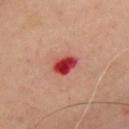Assessment:
Imaged during a routine full-body skin examination; the lesion was not biopsied and no histopathology is available.
Clinical summary:
A male patient, approximately 50 years of age. The lesion-visualizer software estimated border irregularity of about 1.5 on a 0–10 scale, a within-lesion color-variation index near 5.5/10, and a peripheral color-asymmetry measure near 1.5. And it measured a nevus-likeness score of about 0/100. A 15 mm crop from a total-body photograph taken for skin-cancer surveillance. On the chest. The recorded lesion diameter is about 3.5 mm.